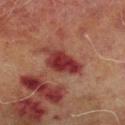No biopsy was performed on this lesion — it was imaged during a full skin examination and was not determined to be concerning. The lesion is located on the left lower leg. A roughly 15 mm field-of-view crop from a total-body skin photograph. This is a cross-polarized tile. A male subject aged approximately 70.